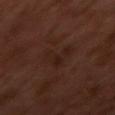The patient is a male aged 28–32.
A 15 mm crop from a total-body photograph taken for skin-cancer surveillance.
Automated image analysis of the tile measured a lesion color around L≈20 a*≈17 b*≈20 in CIELAB and a lesion-to-skin contrast of about 5.5 (normalized; higher = more distinct). And it measured a nevus-likeness score of about 15/100.
Located on the left arm.